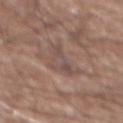Findings:
– workup — catalogued during a skin exam; not biopsied
– lesion diameter — ~4.5 mm (longest diameter)
– image-analysis metrics — a lesion area of about 7 mm² and a shape eccentricity near 0.85; a lesion color around L≈49 a*≈16 b*≈21 in CIELAB, roughly 6 lightness units darker than nearby skin, and a lesion-to-skin contrast of about 5.5 (normalized; higher = more distinct); a border-irregularity rating of about 8/10 and a color-variation rating of about 4.5/10; a nevus-likeness score of about 0/100 and a lesion-detection confidence of about 85/100
– subject — male, about 75 years old
– illumination — white-light
– body site — the abdomen
– imaging modality — ~15 mm tile from a whole-body skin photo